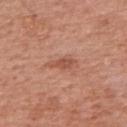Findings:
• workup · imaged on a skin check; not biopsied
• body site · the right upper arm
• lesion diameter · about 3 mm
• patient · female, aged 58–62
• automated metrics · an area of roughly 3.5 mm² and an outline eccentricity of about 0.85 (0 = round, 1 = elongated); a border-irregularity index near 3/10, a color-variation rating of about 1.5/10, and peripheral color asymmetry of about 0.5
• imaging modality · 15 mm crop, total-body photography
• lighting · white-light illumination